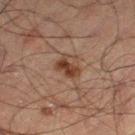{
  "biopsy_status": "not biopsied; imaged during a skin examination",
  "lighting": "cross-polarized",
  "automated_metrics": {
    "border_irregularity_0_10": 3.5,
    "color_variation_0_10": 3.0,
    "peripheral_color_asymmetry": 1.0,
    "nevus_likeness_0_100": 75,
    "lesion_detection_confidence_0_100": 100
  },
  "image": {
    "source": "total-body photography crop",
    "field_of_view_mm": 15
  },
  "site": "left thigh",
  "patient": {
    "sex": "male",
    "age_approx": 50
  },
  "lesion_size": {
    "long_diameter_mm_approx": 3.0
  }
}